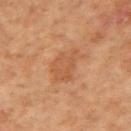Case summary:
– workup — total-body-photography surveillance lesion; no biopsy
– patient — female, approximately 65 years of age
– image source — ~15 mm crop, total-body skin-cancer survey
– automated lesion analysis — a lesion area of about 10 mm², a shape eccentricity near 0.8, and a symmetry-axis asymmetry near 0.3; a border-irregularity index near 4/10, internal color variation of about 2.5 on a 0–10 scale, and a peripheral color-asymmetry measure near 1; an automated nevus-likeness rating near 0 out of 100 and a lesion-detection confidence of about 100/100
– site — the left arm
– size — about 4.5 mm
– lighting — cross-polarized illumination